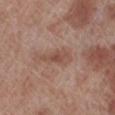notes = imaged on a skin check; not biopsied
image-analysis metrics = border irregularity of about 3.5 on a 0–10 scale, internal color variation of about 2.5 on a 0–10 scale, and a peripheral color-asymmetry measure near 0.5; a nevus-likeness score of about 0/100
anatomic site = the left lower leg
lighting = white-light illumination
acquisition = total-body-photography crop, ~15 mm field of view
lesion diameter = ~4 mm (longest diameter)
patient = male, in their 70s